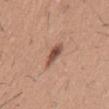biopsy status: catalogued during a skin exam; not biopsied | subject: male, roughly 60 years of age | illumination: white-light | lesion diameter: ~3.5 mm (longest diameter) | acquisition: total-body-photography crop, ~15 mm field of view | TBP lesion metrics: internal color variation of about 4 on a 0–10 scale and radial color variation of about 1; a nevus-likeness score of about 90/100 and lesion-presence confidence of about 100/100 | body site: the lower back.